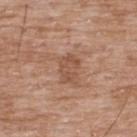The patient is a male roughly 50 years of age. Longest diameter approximately 3 mm. Cropped from a total-body skin-imaging series; the visible field is about 15 mm. On the upper back.